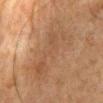{
  "biopsy_status": "not biopsied; imaged during a skin examination",
  "automated_metrics": {
    "area_mm2_approx": 31.0,
    "eccentricity": 0.9,
    "shape_asymmetry": 0.45,
    "cielab_L": 40,
    "cielab_a": 15,
    "cielab_b": 26,
    "vs_skin_darker_L": 5.0,
    "border_irregularity_0_10": 6.5,
    "peripheral_color_asymmetry": 1.0,
    "nevus_likeness_0_100": 0,
    "lesion_detection_confidence_0_100": 95
  },
  "site": "abdomen",
  "image": {
    "source": "total-body photography crop",
    "field_of_view_mm": 15
  },
  "lighting": "cross-polarized",
  "patient": {
    "sex": "male",
    "age_approx": 80
  },
  "lesion_size": {
    "long_diameter_mm_approx": 9.0
  }
}